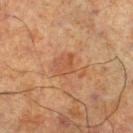Background: The lesion is located on the right lower leg. A lesion tile, about 15 mm wide, cut from a 3D total-body photograph. This is a cross-polarized tile. A male subject, aged approximately 70. The lesion-visualizer software estimated a detector confidence of about 100 out of 100 that the crop contains a lesion. The recorded lesion diameter is about 4 mm.